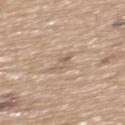{
  "biopsy_status": "not biopsied; imaged during a skin examination",
  "lighting": "white-light",
  "image": {
    "source": "total-body photography crop",
    "field_of_view_mm": 15
  },
  "automated_metrics": {
    "area_mm2_approx": 2.5,
    "eccentricity": 0.85,
    "shape_asymmetry": 0.35,
    "cielab_L": 60,
    "cielab_a": 15,
    "cielab_b": 27,
    "vs_skin_contrast_norm": 5.0,
    "border_irregularity_0_10": 4.0,
    "color_variation_0_10": 0.0,
    "peripheral_color_asymmetry": 0.0
  },
  "site": "upper back",
  "patient": {
    "sex": "male",
    "age_approx": 65
  }
}